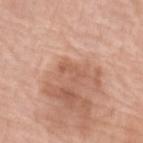follow-up: imaged on a skin check; not biopsied | anatomic site: the left forearm | subject: female, approximately 70 years of age | imaging modality: ~15 mm crop, total-body skin-cancer survey | automated lesion analysis: a lesion area of about 2.5 mm², an outline eccentricity of about 0.9 (0 = round, 1 = elongated), and a symmetry-axis asymmetry near 0.65; an automated nevus-likeness rating near 0 out of 100 and a detector confidence of about 100 out of 100 that the crop contains a lesion | lighting: white-light illumination.